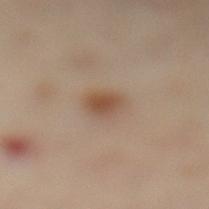An algorithmic analysis of the crop reported an average lesion color of about L≈43 a*≈14 b*≈25 (CIELAB), about 8 CIELAB-L* units darker than the surrounding skin, and a normalized lesion–skin contrast near 7. And it measured a border-irregularity index near 1.5/10, internal color variation of about 4 on a 0–10 scale, and radial color variation of about 1.5. The software also gave a nevus-likeness score of about 95/100 and a lesion-detection confidence of about 100/100. A female patient, aged around 60. This is a cross-polarized tile. Cropped from a total-body skin-imaging series; the visible field is about 15 mm. Approximately 3 mm at its widest. From the leg.